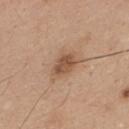biopsy status — total-body-photography surveillance lesion; no biopsy | acquisition — 15 mm crop, total-body photography | size — ~3 mm (longest diameter) | patient — male, aged 33 to 37 | illumination — white-light | body site — the mid back.